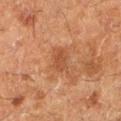Acquisition and patient details:
Cropped from a total-body skin-imaging series; the visible field is about 15 mm. About 2.5 mm across. A female patient, aged 48–52. This is a cross-polarized tile. The lesion is located on the left lower leg. The total-body-photography lesion software estimated a lesion color around L≈40 a*≈22 b*≈31 in CIELAB and a normalized lesion–skin contrast near 6. It also reported border irregularity of about 2.5 on a 0–10 scale, a within-lesion color-variation index near 2/10, and radial color variation of about 0.5.